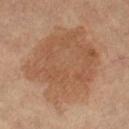– subject · female, aged around 70
– size · ~9.5 mm (longest diameter)
– location · the leg
– lighting · cross-polarized illumination
– image · 15 mm crop, total-body photography